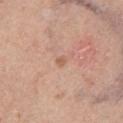Part of a total-body skin-imaging series; this lesion was reviewed on a skin check and was not flagged for biopsy.
The subject is a male aged 48 to 52.
A close-up tile cropped from a whole-body skin photograph, about 15 mm across.
Approximately 1 mm at its widest.
The lesion is on the chest.